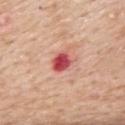Imaged during a routine full-body skin examination; the lesion was not biopsied and no histopathology is available.
A female subject in their 70s.
A 15 mm close-up tile from a total-body photography series done for melanoma screening.
Longest diameter approximately 2.5 mm.
An algorithmic analysis of the crop reported an eccentricity of roughly 0.55 and a symmetry-axis asymmetry near 0.15. It also reported a mean CIELAB color near L≈51 a*≈40 b*≈27, roughly 18 lightness units darker than nearby skin, and a normalized lesion–skin contrast near 12. The software also gave a classifier nevus-likeness of about 0/100 and a lesion-detection confidence of about 100/100.
From the upper back.
The tile uses white-light illumination.